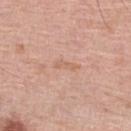Findings:
* workup — catalogued during a skin exam; not biopsied
* subject — male, aged approximately 80
* site — the right lower leg
* image — ~15 mm crop, total-body skin-cancer survey